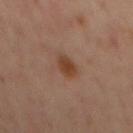Q: Is there a histopathology result?
A: imaged on a skin check; not biopsied
Q: Who is the patient?
A: male, approximately 65 years of age
Q: Where on the body is the lesion?
A: the mid back
Q: What kind of image is this?
A: total-body-photography crop, ~15 mm field of view
Q: Illumination type?
A: cross-polarized
Q: What did automated image analysis measure?
A: a lesion color around L≈39 a*≈19 b*≈29 in CIELAB, roughly 9 lightness units darker than nearby skin, and a normalized border contrast of about 9; a classifier nevus-likeness of about 85/100 and a detector confidence of about 100 out of 100 that the crop contains a lesion